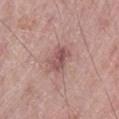Q: Was a biopsy performed?
A: catalogued during a skin exam; not biopsied
Q: Lesion location?
A: the leg
Q: Who is the patient?
A: male, about 70 years old
Q: What kind of image is this?
A: total-body-photography crop, ~15 mm field of view
Q: How was the tile lit?
A: white-light illumination